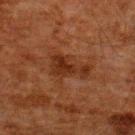Q: Was this lesion biopsied?
A: no biopsy performed (imaged during a skin exam)
Q: Where on the body is the lesion?
A: the back
Q: How large is the lesion?
A: ~4.5 mm (longest diameter)
Q: What is the imaging modality?
A: total-body-photography crop, ~15 mm field of view
Q: What did automated image analysis measure?
A: a lesion area of about 10 mm² and a shape-asymmetry score of about 0.5 (0 = symmetric); border irregularity of about 6.5 on a 0–10 scale, internal color variation of about 3 on a 0–10 scale, and peripheral color asymmetry of about 1
Q: Who is the patient?
A: male, aged 58–62
Q: How was the tile lit?
A: cross-polarized